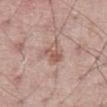Assessment:
The lesion was photographed on a routine skin check and not biopsied; there is no pathology result.
Clinical summary:
A male subject aged approximately 65. From the abdomen. This image is a 15 mm lesion crop taken from a total-body photograph. Captured under white-light illumination. The total-body-photography lesion software estimated a normalized border contrast of about 6.5. The analysis additionally found a border-irregularity index near 5/10.